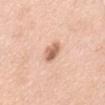A male subject, aged approximately 60. About 3.5 mm across. Automated tile analysis of the lesion measured a lesion area of about 5 mm² and an outline eccentricity of about 0.85 (0 = round, 1 = elongated). The software also gave a within-lesion color-variation index near 5/10 and peripheral color asymmetry of about 2. It also reported an automated nevus-likeness rating near 95 out of 100. The lesion is on the abdomen. A roughly 15 mm field-of-view crop from a total-body skin photograph. The tile uses white-light illumination.